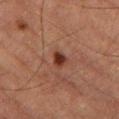The lesion was photographed on a routine skin check and not biopsied; there is no pathology result. Located on the left thigh. A 15 mm close-up tile from a total-body photography series done for melanoma screening. The tile uses cross-polarized illumination. The subject is a male aged around 85 or older. Automated tile analysis of the lesion measured an area of roughly 4 mm² and an outline eccentricity of about 0.6 (0 = round, 1 = elongated). And it measured border irregularity of about 3 on a 0–10 scale and a color-variation rating of about 3.5/10.